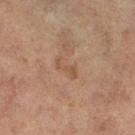The lesion's longest dimension is about 3 mm. This image is a 15 mm lesion crop taken from a total-body photograph. Captured under cross-polarized illumination. Located on the right thigh. A female subject, aged 58–62.